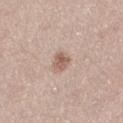A close-up tile cropped from a whole-body skin photograph, about 15 mm across. The lesion is located on the right thigh. A female subject aged 48 to 52.